Cropped from a total-body skin-imaging series; the visible field is about 15 mm; about 3 mm across; the patient is a female in their mid-60s; from the left upper arm; this is a white-light tile:
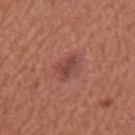On excision, pathology confirmed a dysplastic (Clark) nevus.